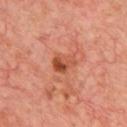Imaged during a routine full-body skin examination; the lesion was not biopsied and no histopathology is available.
From the chest.
Imaged with cross-polarized lighting.
An algorithmic analysis of the crop reported an average lesion color of about L≈49 a*≈29 b*≈35 (CIELAB), about 11 CIELAB-L* units darker than the surrounding skin, and a lesion-to-skin contrast of about 8 (normalized; higher = more distinct). The software also gave a lesion-detection confidence of about 100/100.
About 3 mm across.
A male subject, in their mid-60s.
A 15 mm close-up extracted from a 3D total-body photography capture.